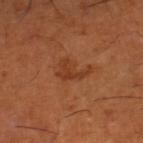Q: Was this lesion biopsied?
A: no biopsy performed (imaged during a skin exam)
Q: What is the imaging modality?
A: total-body-photography crop, ~15 mm field of view
Q: What is the anatomic site?
A: the right lower leg
Q: Illumination type?
A: cross-polarized
Q: Automated lesion metrics?
A: a lesion color around L≈39 a*≈26 b*≈35 in CIELAB, about 7 CIELAB-L* units darker than the surrounding skin, and a lesion-to-skin contrast of about 6 (normalized; higher = more distinct)
Q: What are the patient's age and sex?
A: male, approximately 65 years of age